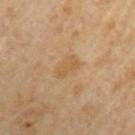A 15 mm close-up tile from a total-body photography series done for melanoma screening. The subject is a male roughly 70 years of age. Located on the arm. Longest diameter approximately 3 mm.